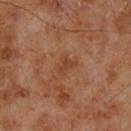Q: Was this lesion biopsied?
A: imaged on a skin check; not biopsied
Q: What is the lesion's diameter?
A: ≈2.5 mm
Q: What kind of image is this?
A: ~15 mm tile from a whole-body skin photo
Q: Lesion location?
A: the left lower leg
Q: Who is the patient?
A: male, aged 68 to 72
Q: Automated lesion metrics?
A: a footprint of about 3.5 mm², an outline eccentricity of about 0.7 (0 = round, 1 = elongated), and two-axis asymmetry of about 0.4; a nevus-likeness score of about 0/100 and a lesion-detection confidence of about 100/100